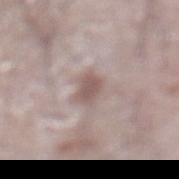workup = no biopsy performed (imaged during a skin exam) | lighting = white-light illumination | lesion size = about 3 mm | automated metrics = a footprint of about 5 mm², a shape eccentricity near 0.6, and a symmetry-axis asymmetry near 0.25; a lesion color around L≈55 a*≈16 b*≈19 in CIELAB, about 10 CIELAB-L* units darker than the surrounding skin, and a normalized border contrast of about 7; a border-irregularity rating of about 2.5/10 and a color-variation rating of about 1.5/10; lesion-presence confidence of about 70/100 | anatomic site = the abdomen | subject = male, aged approximately 75 | imaging modality = 15 mm crop, total-body photography.